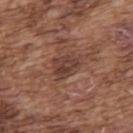No biopsy was performed on this lesion — it was imaged during a full skin examination and was not determined to be concerning.
Longest diameter approximately 3.5 mm.
A male patient aged 73 to 77.
The tile uses white-light illumination.
A close-up tile cropped from a whole-body skin photograph, about 15 mm across.
From the upper back.